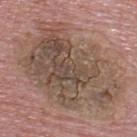| feature | finding |
|---|---|
| notes | total-body-photography surveillance lesion; no biopsy |
| anatomic site | the upper back |
| image-analysis metrics | a lesion area of about 80 mm², a shape eccentricity near 0.7, and a shape-asymmetry score of about 0.2 (0 = symmetric) |
| acquisition | 15 mm crop, total-body photography |
| patient | male, aged 73–77 |
| lesion size | ≈12.5 mm |
| lighting | white-light illumination |